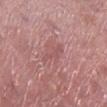The recorded lesion diameter is about 4 mm.
The subject is a male aged approximately 70.
From the right lower leg.
A 15 mm close-up extracted from a 3D total-body photography capture.
This is a white-light tile.
Automated image analysis of the tile measured a footprint of about 3 mm², an outline eccentricity of about 0.95 (0 = round, 1 = elongated), and a symmetry-axis asymmetry near 0.45. The software also gave border irregularity of about 6 on a 0–10 scale and a color-variation rating of about 0/10.